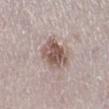tile lighting = white-light
image = total-body-photography crop, ~15 mm field of view
body site = the right lower leg
patient = female, aged 48–52
lesion size = about 4 mm